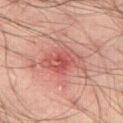No biopsy was performed on this lesion — it was imaged during a full skin examination and was not determined to be concerning.
Cropped from a whole-body photographic skin survey; the tile spans about 15 mm.
Located on the right thigh.
A male patient, roughly 35 years of age.
Imaged with cross-polarized lighting.
Measured at roughly 5 mm in maximum diameter.